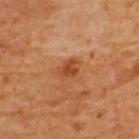Assessment: This lesion was catalogued during total-body skin photography and was not selected for biopsy. Background: The lesion is on the upper back. The subject is a male aged around 65. Cropped from a whole-body photographic skin survey; the tile spans about 15 mm. Imaged with cross-polarized lighting.